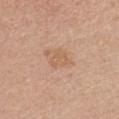{"biopsy_status": "not biopsied; imaged during a skin examination", "image": {"source": "total-body photography crop", "field_of_view_mm": 15}, "lesion_size": {"long_diameter_mm_approx": 2.5}, "patient": {"sex": "female", "age_approx": 45}, "site": "front of the torso", "lighting": "white-light"}